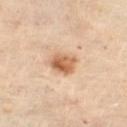Impression: Imaged during a routine full-body skin examination; the lesion was not biopsied and no histopathology is available. Background: Imaged with cross-polarized lighting. The patient is a female aged around 60. A region of skin cropped from a whole-body photographic capture, roughly 15 mm wide. The lesion is located on the right thigh. Automated image analysis of the tile measured a footprint of about 6.5 mm², an outline eccentricity of about 0.5 (0 = round, 1 = elongated), and two-axis asymmetry of about 0.25. It also reported an average lesion color of about L≈61 a*≈22 b*≈36 (CIELAB), roughly 14 lightness units darker than nearby skin, and a normalized border contrast of about 9. It also reported border irregularity of about 2.5 on a 0–10 scale and radial color variation of about 2.5. Measured at roughly 3 mm in maximum diameter.